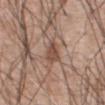Q: Is there a histopathology result?
A: no biopsy performed (imaged during a skin exam)
Q: Automated lesion metrics?
A: an area of roughly 5.5 mm² and an eccentricity of roughly 0.75; a mean CIELAB color near L≈49 a*≈19 b*≈26 and a normalized lesion–skin contrast near 7.5; a within-lesion color-variation index near 3/10 and peripheral color asymmetry of about 1; a nevus-likeness score of about 5/100 and lesion-presence confidence of about 100/100
Q: What are the patient's age and sex?
A: male, aged 58 to 62
Q: What kind of image is this?
A: 15 mm crop, total-body photography
Q: Illumination type?
A: white-light
Q: Lesion location?
A: the abdomen
Q: How large is the lesion?
A: ≈3 mm